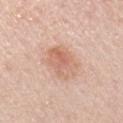The lesion was tiled from a total-body skin photograph and was not biopsied. Located on the left upper arm. The total-body-photography lesion software estimated a border-irregularity rating of about 3/10, a within-lesion color-variation index near 4.5/10, and peripheral color asymmetry of about 1.5. The software also gave a classifier nevus-likeness of about 50/100 and a lesion-detection confidence of about 100/100. About 4.5 mm across. A 15 mm close-up tile from a total-body photography series done for melanoma screening. The patient is a male approximately 60 years of age.